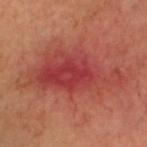Clinical impression: No biopsy was performed on this lesion — it was imaged during a full skin examination and was not determined to be concerning. Clinical summary: Imaged with cross-polarized lighting. Longest diameter approximately 11 mm. A male patient, approximately 45 years of age. The lesion is on the head or neck. A roughly 15 mm field-of-view crop from a total-body skin photograph.